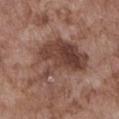Part of a total-body skin-imaging series; this lesion was reviewed on a skin check and was not flagged for biopsy.
Imaged with white-light lighting.
On the abdomen.
Automated tile analysis of the lesion measured an eccentricity of roughly 0.75 and a symmetry-axis asymmetry near 0.45. It also reported a lesion color around L≈42 a*≈19 b*≈24 in CIELAB, about 11 CIELAB-L* units darker than the surrounding skin, and a normalized lesion–skin contrast near 8.5.
A male subject, aged approximately 75.
A 15 mm close-up extracted from a 3D total-body photography capture.